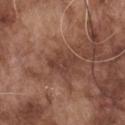Assessment: The lesion was tiled from a total-body skin photograph and was not biopsied. Clinical summary: The subject is a male aged approximately 75. A close-up tile cropped from a whole-body skin photograph, about 15 mm across. From the chest.